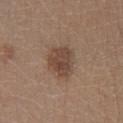Recorded during total-body skin imaging; not selected for excision or biopsy. The lesion is located on the left lower leg. Longest diameter approximately 4 mm. This image is a 15 mm lesion crop taken from a total-body photograph. The tile uses white-light illumination. Automated image analysis of the tile measured a nevus-likeness score of about 40/100 and a detector confidence of about 100 out of 100 that the crop contains a lesion. A female patient, approximately 40 years of age.